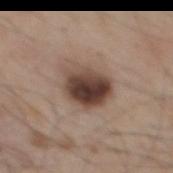Notes:
- biopsy status: no biopsy performed (imaged during a skin exam)
- patient: male, aged 43–47
- automated metrics: a lesion area of about 15 mm², an outline eccentricity of about 0.6 (0 = round, 1 = elongated), and a shape-asymmetry score of about 0.2 (0 = symmetric); a mean CIELAB color near L≈42 a*≈16 b*≈23, roughly 16 lightness units darker than nearby skin, and a lesion-to-skin contrast of about 12 (normalized; higher = more distinct); internal color variation of about 9 on a 0–10 scale and a peripheral color-asymmetry measure near 4
- acquisition: ~15 mm tile from a whole-body skin photo
- location: the mid back
- tile lighting: white-light illumination